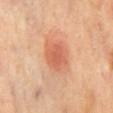Clinical impression:
The lesion was tiled from a total-body skin photograph and was not biopsied.
Clinical summary:
Automated tile analysis of the lesion measured a footprint of about 9 mm², an outline eccentricity of about 0.5 (0 = round, 1 = elongated), and two-axis asymmetry of about 0.2. The software also gave a border-irregularity index near 2/10, a color-variation rating of about 2/10, and peripheral color asymmetry of about 0.5. The software also gave a lesion-detection confidence of about 100/100. The lesion's longest dimension is about 3.5 mm. A 15 mm crop from a total-body photograph taken for skin-cancer surveillance. From the mid back. The tile uses cross-polarized illumination.